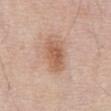Imaged during a routine full-body skin examination; the lesion was not biopsied and no histopathology is available. A male patient, about 70 years old. The recorded lesion diameter is about 4 mm. Automated image analysis of the tile measured an area of roughly 9.5 mm², an outline eccentricity of about 0.8 (0 = round, 1 = elongated), and two-axis asymmetry of about 0.2. And it measured a nevus-likeness score of about 75/100. Captured under white-light illumination. Cropped from a total-body skin-imaging series; the visible field is about 15 mm. The lesion is located on the abdomen.